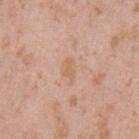Captured during whole-body skin photography for melanoma surveillance; the lesion was not biopsied. A male patient about 40 years old. A 15 mm close-up tile from a total-body photography series done for melanoma screening. Longest diameter approximately 2.5 mm. The tile uses white-light illumination. The lesion is on the left upper arm.